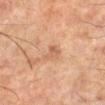  biopsy_status: not biopsied; imaged during a skin examination
  patient:
    sex: male
    age_approx: 60
  site: leg
  image:
    source: total-body photography crop
    field_of_view_mm: 15
  lighting: cross-polarized